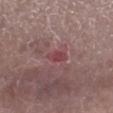The lesion was tiled from a total-body skin photograph and was not biopsied. The patient is a female aged 73–77. Cropped from a whole-body photographic skin survey; the tile spans about 15 mm. From the right lower leg. This is a white-light tile. Measured at roughly 2.5 mm in maximum diameter.